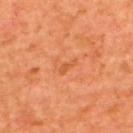biopsy status: catalogued during a skin exam; not biopsied | image-analysis metrics: an average lesion color of about L≈56 a*≈31 b*≈44 (CIELAB), about 7 CIELAB-L* units darker than the surrounding skin, and a lesion-to-skin contrast of about 5 (normalized; higher = more distinct); a border-irregularity index near 4.5/10, a within-lesion color-variation index near 0/10, and radial color variation of about 0 | body site: the upper back | lesion size: ~2.5 mm (longest diameter) | lighting: cross-polarized illumination | patient: male, in their mid- to late 60s | image source: ~15 mm tile from a whole-body skin photo.